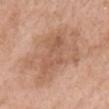Q: Was a biopsy performed?
A: imaged on a skin check; not biopsied
Q: What are the patient's age and sex?
A: female, about 70 years old
Q: What is the anatomic site?
A: the head or neck
Q: What is the imaging modality?
A: ~15 mm tile from a whole-body skin photo
Q: Illumination type?
A: white-light
Q: How large is the lesion?
A: ~9 mm (longest diameter)
Q: What did automated image analysis measure?
A: an average lesion color of about L≈59 a*≈20 b*≈31 (CIELAB) and a lesion–skin lightness drop of about 8; a border-irregularity rating of about 5.5/10, a within-lesion color-variation index near 4/10, and peripheral color asymmetry of about 1.5; a nevus-likeness score of about 5/100